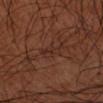The lesion was photographed on a routine skin check and not biopsied; there is no pathology result. Captured under cross-polarized illumination. Automated tile analysis of the lesion measured about 5 CIELAB-L* units darker than the surrounding skin and a lesion-to-skin contrast of about 5.5 (normalized; higher = more distinct). From the left forearm. A male subject, aged 58 to 62. This image is a 15 mm lesion crop taken from a total-body photograph.